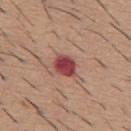This lesion was catalogued during total-body skin photography and was not selected for biopsy.
The lesion is on the mid back.
A male subject aged 58 to 62.
Cropped from a whole-body photographic skin survey; the tile spans about 15 mm.
Automated tile analysis of the lesion measured internal color variation of about 4 on a 0–10 scale and a peripheral color-asymmetry measure near 1.5. The software also gave a classifier nevus-likeness of about 0/100 and a lesion-detection confidence of about 100/100.
Approximately 3 mm at its widest.